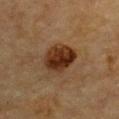Background:
A male patient in their mid-80s or older. On the chest. Cropped from a total-body skin-imaging series; the visible field is about 15 mm.